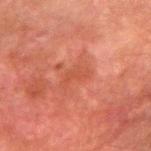Imaged during a routine full-body skin examination; the lesion was not biopsied and no histopathology is available. The total-body-photography lesion software estimated a lesion color around L≈41 a*≈26 b*≈29 in CIELAB and roughly 5 lightness units darker than nearby skin. And it measured border irregularity of about 4.5 on a 0–10 scale, internal color variation of about 1 on a 0–10 scale, and a peripheral color-asymmetry measure near 0.5. Cropped from a whole-body photographic skin survey; the tile spans about 15 mm. A male subject aged around 80. Captured under cross-polarized illumination. The lesion is on the right forearm. The recorded lesion diameter is about 3.5 mm.